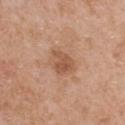The lesion was photographed on a routine skin check and not biopsied; there is no pathology result. The lesion is on the chest. A roughly 15 mm field-of-view crop from a total-body skin photograph. A female patient in their 40s. Imaged with white-light lighting.